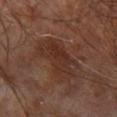The lesion was tiled from a total-body skin photograph and was not biopsied. The patient is a male aged 58–62. Cropped from a whole-body photographic skin survey; the tile spans about 15 mm. The lesion is on the right leg. Captured under cross-polarized illumination.